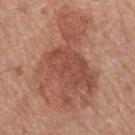Q: What is the lesion's diameter?
A: about 11.5 mm
Q: Who is the patient?
A: female, roughly 50 years of age
Q: Lesion location?
A: the left forearm
Q: How was this image acquired?
A: ~15 mm crop, total-body skin-cancer survey
Q: Illumination type?
A: white-light